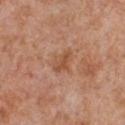Q: Was a biopsy performed?
A: total-body-photography surveillance lesion; no biopsy
Q: How was this image acquired?
A: 15 mm crop, total-body photography
Q: What did automated image analysis measure?
A: a lesion area of about 4.5 mm², an outline eccentricity of about 0.8 (0 = round, 1 = elongated), and a shape-asymmetry score of about 0.25 (0 = symmetric); internal color variation of about 2.5 on a 0–10 scale and a peripheral color-asymmetry measure near 1; a nevus-likeness score of about 0/100 and lesion-presence confidence of about 100/100
Q: Illumination type?
A: white-light illumination
Q: Who is the patient?
A: male, in their mid- to late 60s
Q: Lesion location?
A: the chest